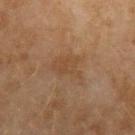{
  "lighting": "cross-polarized",
  "site": "left forearm",
  "patient": {
    "sex": "female",
    "age_approx": 60
  },
  "image": {
    "source": "total-body photography crop",
    "field_of_view_mm": 15
  },
  "lesion_size": {
    "long_diameter_mm_approx": 5.0
  }
}